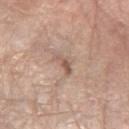workup: imaged on a skin check; not biopsied
subject: female, aged approximately 65
imaging modality: total-body-photography crop, ~15 mm field of view
illumination: white-light illumination
lesion size: ~2.5 mm (longest diameter)
automated lesion analysis: a lesion area of about 2.5 mm², an outline eccentricity of about 0.9 (0 = round, 1 = elongated), and a symmetry-axis asymmetry near 0.5; border irregularity of about 5.5 on a 0–10 scale and radial color variation of about 0
anatomic site: the left forearm